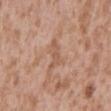biopsy status: imaged on a skin check; not biopsied | location: the back | lighting: white-light illumination | patient: male, about 45 years old | imaging modality: 15 mm crop, total-body photography | diameter: about 3.5 mm.